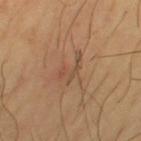follow-up: imaged on a skin check; not biopsied
diameter: ≈3.5 mm
tile lighting: cross-polarized
location: the left thigh
acquisition: 15 mm crop, total-body photography
patient: male, aged 63–67